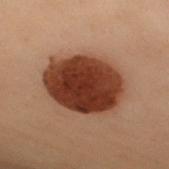Part of a total-body skin-imaging series; this lesion was reviewed on a skin check and was not flagged for biopsy. A 15 mm close-up extracted from a 3D total-body photography capture. The tile uses cross-polarized illumination. Measured at roughly 8 mm in maximum diameter. A female patient, aged approximately 60. The lesion-visualizer software estimated a lesion–skin lightness drop of about 18 and a normalized lesion–skin contrast near 15. The lesion is on the back.